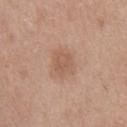The patient is a male roughly 50 years of age. Cropped from a total-body skin-imaging series; the visible field is about 15 mm. Measured at roughly 3 mm in maximum diameter. Captured under white-light illumination. Automated tile analysis of the lesion measured an outline eccentricity of about 0.65 (0 = round, 1 = elongated) and two-axis asymmetry of about 0.3. The software also gave a border-irregularity index near 3/10, a color-variation rating of about 2/10, and a peripheral color-asymmetry measure near 1. The lesion is located on the abdomen.